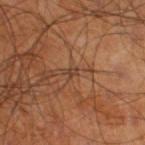biopsy_status: not biopsied; imaged during a skin examination
automated_metrics:
  area_mm2_approx: 1.0
  shape_asymmetry: 0.25
  nevus_likeness_0_100: 0
lighting: cross-polarized
image:
  source: total-body photography crop
  field_of_view_mm: 15
site: right thigh
lesion_size:
  long_diameter_mm_approx: 1.0
patient:
  sex: male
  age_approx: 60